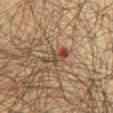This lesion was catalogued during total-body skin photography and was not selected for biopsy.
The lesion's longest dimension is about 4 mm.
On the mid back.
A 15 mm close-up tile from a total-body photography series done for melanoma screening.
The patient is a male aged approximately 50.
Captured under cross-polarized illumination.
The total-body-photography lesion software estimated a lesion–skin lightness drop of about 10. And it measured a border-irregularity index near 5.5/10, internal color variation of about 6.5 on a 0–10 scale, and radial color variation of about 1.